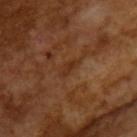This image is a 15 mm lesion crop taken from a total-body photograph. An algorithmic analysis of the crop reported a lesion color around L≈30 a*≈21 b*≈31 in CIELAB and a lesion–skin lightness drop of about 6. And it measured peripheral color asymmetry of about 0. And it measured an automated nevus-likeness rating near 0 out of 100 and a detector confidence of about 75 out of 100 that the crop contains a lesion. Imaged with cross-polarized lighting. A male subject approximately 65 years of age.